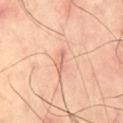notes=imaged on a skin check; not biopsied
acquisition=15 mm crop, total-body photography
site=the right thigh
lesion diameter=about 3 mm
lighting=cross-polarized